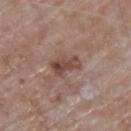Case summary:
- notes — no biopsy performed (imaged during a skin exam)
- site — the upper back
- image source — total-body-photography crop, ~15 mm field of view
- patient — male, aged 63–67